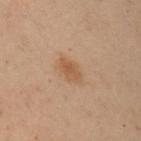Assessment:
Part of a total-body skin-imaging series; this lesion was reviewed on a skin check and was not flagged for biopsy.
Acquisition and patient details:
A 15 mm crop from a total-body photograph taken for skin-cancer surveillance. Captured under cross-polarized illumination. On the right upper arm. The subject is a male aged 53 to 57. The recorded lesion diameter is about 3 mm.